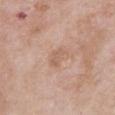<tbp_lesion>
  <lesion_size>
    <long_diameter_mm_approx>2.5</long_diameter_mm_approx>
  </lesion_size>
  <site>chest</site>
  <image>
    <source>total-body photography crop</source>
    <field_of_view_mm>15</field_of_view_mm>
  </image>
  <lighting>white-light</lighting>
  <patient>
    <sex>female</sex>
    <age_approx>70</age_approx>
  </patient>
</tbp_lesion>